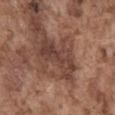Recorded during total-body skin imaging; not selected for excision or biopsy.
Imaged with white-light lighting.
Longest diameter approximately 6.5 mm.
Located on the abdomen.
A 15 mm close-up tile from a total-body photography series done for melanoma screening.
A male patient in their mid- to late 70s.